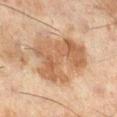Assessment: No biopsy was performed on this lesion — it was imaged during a full skin examination and was not determined to be concerning. Image and clinical context: About 7 mm across. The tile uses cross-polarized illumination. The lesion is located on the right lower leg. A close-up tile cropped from a whole-body skin photograph, about 15 mm across. Automated image analysis of the tile measured an outline eccentricity of about 0.6 (0 = round, 1 = elongated) and a symmetry-axis asymmetry near 0.35. The analysis additionally found a lesion color around L≈48 a*≈16 b*≈29 in CIELAB and about 9 CIELAB-L* units darker than the surrounding skin. A male subject, in their mid-40s.